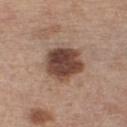Notes:
– workup — catalogued during a skin exam; not biopsied
– acquisition — 15 mm crop, total-body photography
– location — the chest
– tile lighting — white-light
– lesion diameter — ≈4.5 mm
– patient — female, in their mid-40s